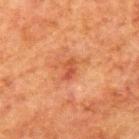Impression:
Part of a total-body skin-imaging series; this lesion was reviewed on a skin check and was not flagged for biopsy.
Acquisition and patient details:
A male patient roughly 80 years of age. The tile uses cross-polarized illumination. A close-up tile cropped from a whole-body skin photograph, about 15 mm across. The lesion is located on the mid back. The recorded lesion diameter is about 2.5 mm.